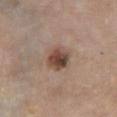| key | value |
|---|---|
| follow-up | no biopsy performed (imaged during a skin exam) |
| acquisition | ~15 mm crop, total-body skin-cancer survey |
| subject | female, aged 63–67 |
| anatomic site | the chest |